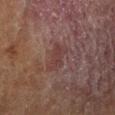Assessment:
No biopsy was performed on this lesion — it was imaged during a full skin examination and was not determined to be concerning.
Acquisition and patient details:
The patient is a male about 85 years old or older. Located on the abdomen. Imaged with cross-polarized lighting. A 15 mm close-up extracted from a 3D total-body photography capture. The recorded lesion diameter is about 4 mm.